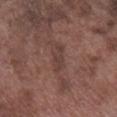| key | value |
|---|---|
| follow-up | no biopsy performed (imaged during a skin exam) |
| image source | total-body-photography crop, ~15 mm field of view |
| tile lighting | white-light |
| size | ≈3.5 mm |
| body site | the leg |
| patient | male, aged 73–77 |